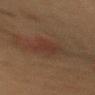site: the mid back
TBP lesion metrics: a footprint of about 7.5 mm²; a mean CIELAB color near L≈29 a*≈17 b*≈22 and a lesion–skin lightness drop of about 5
subject: female, about 40 years old
lighting: cross-polarized
imaging modality: total-body-photography crop, ~15 mm field of view
size: ≈4.5 mm